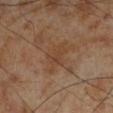No biopsy was performed on this lesion — it was imaged during a full skin examination and was not determined to be concerning. A male patient roughly 70 years of age. The lesion is on the left lower leg. Cropped from a whole-body photographic skin survey; the tile spans about 15 mm.